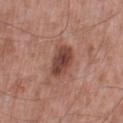Clinical impression: Part of a total-body skin-imaging series; this lesion was reviewed on a skin check and was not flagged for biopsy. Clinical summary: Measured at roughly 4 mm in maximum diameter. A male patient, aged approximately 55. Cropped from a total-body skin-imaging series; the visible field is about 15 mm. The tile uses white-light illumination. The lesion is on the leg.